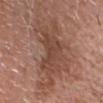notes: total-body-photography surveillance lesion; no biopsy
anatomic site: the chest
acquisition: ~15 mm crop, total-body skin-cancer survey
lesion diameter: ~10.5 mm (longest diameter)
lighting: white-light illumination
subject: male, about 80 years old